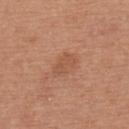The lesion was tiled from a total-body skin photograph and was not biopsied. Measured at roughly 3.5 mm in maximum diameter. A 15 mm crop from a total-body photograph taken for skin-cancer surveillance. This is a white-light tile. The lesion is located on the back. A male subject about 65 years old.